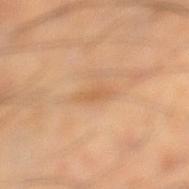The lesion was tiled from a total-body skin photograph and was not biopsied. Automated tile analysis of the lesion measured a lesion area of about 3 mm², an outline eccentricity of about 0.9 (0 = round, 1 = elongated), and a shape-asymmetry score of about 0.3 (0 = symmetric). The software also gave about 7 CIELAB-L* units darker than the surrounding skin and a normalized border contrast of about 5.5. And it measured a border-irregularity index near 3.5/10 and radial color variation of about 0.5. The analysis additionally found an automated nevus-likeness rating near 0 out of 100 and a detector confidence of about 100 out of 100 that the crop contains a lesion. On the left lower leg. A male patient, approximately 40 years of age. A 15 mm crop from a total-body photograph taken for skin-cancer surveillance. The recorded lesion diameter is about 3 mm. The tile uses cross-polarized illumination.